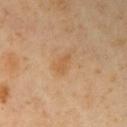{"biopsy_status": "not biopsied; imaged during a skin examination", "lighting": "cross-polarized", "automated_metrics": {"cielab_L": 56, "cielab_a": 19, "cielab_b": 38, "vs_skin_contrast_norm": 5.5, "nevus_likeness_0_100": 10, "lesion_detection_confidence_0_100": 100}, "image": {"source": "total-body photography crop", "field_of_view_mm": 15}, "site": "right upper arm", "lesion_size": {"long_diameter_mm_approx": 2.5}, "patient": {"sex": "female", "age_approx": 50}}